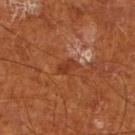Image and clinical context: A 15 mm crop from a total-body photograph taken for skin-cancer surveillance. The subject is a male aged approximately 65. Located on the right lower leg. Captured under cross-polarized illumination.